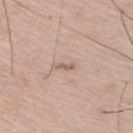Clinical impression:
The lesion was tiled from a total-body skin photograph and was not biopsied.
Acquisition and patient details:
An algorithmic analysis of the crop reported a footprint of about 1.5 mm², an outline eccentricity of about 0.9 (0 = round, 1 = elongated), and a symmetry-axis asymmetry near 0.55. It also reported a mean CIELAB color near L≈60 a*≈17 b*≈27 and a lesion–skin lightness drop of about 9. It also reported a border-irregularity index near 5/10, internal color variation of about 0 on a 0–10 scale, and a peripheral color-asymmetry measure near 0. And it measured a nevus-likeness score of about 0/100 and a lesion-detection confidence of about 95/100. The tile uses white-light illumination. A male patient aged 73–77. Cropped from a total-body skin-imaging series; the visible field is about 15 mm. The lesion is on the upper back. Approximately 2 mm at its widest.